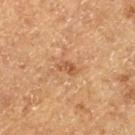notes = total-body-photography surveillance lesion; no biopsy | acquisition = total-body-photography crop, ~15 mm field of view | image-analysis metrics = a classifier nevus-likeness of about 0/100 and lesion-presence confidence of about 100/100 | lighting = cross-polarized | location = the left thigh | lesion diameter = ≈2.5 mm | patient = male, aged 73–77.